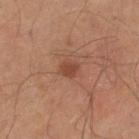Cropped from a total-body skin-imaging series; the visible field is about 15 mm. This is a cross-polarized tile. About 2 mm across. A male subject, aged approximately 60. Located on the right thigh.